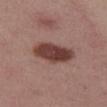Impression: Captured during whole-body skin photography for melanoma surveillance; the lesion was not biopsied. Image and clinical context: Measured at roughly 5 mm in maximum diameter. A female subject, aged approximately 55. A region of skin cropped from a whole-body photographic capture, roughly 15 mm wide. On the leg.